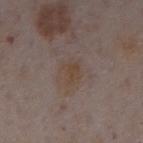follow-up = catalogued during a skin exam; not biopsied.